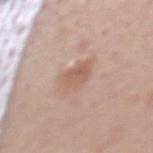Recorded during total-body skin imaging; not selected for excision or biopsy. A 15 mm crop from a total-body photograph taken for skin-cancer surveillance. From the chest. A male subject, in their mid-50s. Captured under white-light illumination.